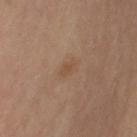{"biopsy_status": "not biopsied; imaged during a skin examination", "lighting": "cross-polarized", "site": "left upper arm", "image": {"source": "total-body photography crop", "field_of_view_mm": 15}, "automated_metrics": {"border_irregularity_0_10": 3.0, "peripheral_color_asymmetry": 0.5}, "patient": {"sex": "female", "age_approx": 65}}